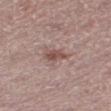Clinical impression: No biopsy was performed on this lesion — it was imaged during a full skin examination and was not determined to be concerning. Context: A roughly 15 mm field-of-view crop from a total-body skin photograph. The subject is a female aged approximately 55. On the leg. The lesion's longest dimension is about 2.5 mm. The tile uses white-light illumination.